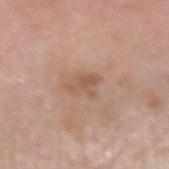Assessment: This lesion was catalogued during total-body skin photography and was not selected for biopsy. Image and clinical context: The patient is a male aged around 80. On the right forearm. A close-up tile cropped from a whole-body skin photograph, about 15 mm across.